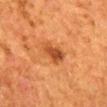biopsy status — catalogued during a skin exam; not biopsied | tile lighting — cross-polarized | patient — female, roughly 50 years of age | location — the mid back | imaging modality — total-body-photography crop, ~15 mm field of view | diameter — ≈3.5 mm.